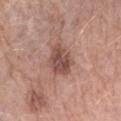Notes:
• workup — total-body-photography surveillance lesion; no biopsy
• illumination — white-light
• automated metrics — a lesion area of about 9 mm², an eccentricity of roughly 0.55, and two-axis asymmetry of about 0.2; a border-irregularity rating of about 2/10 and a color-variation rating of about 3.5/10; a lesion-detection confidence of about 100/100
• lesion diameter — ~4 mm (longest diameter)
• image source — total-body-photography crop, ~15 mm field of view
• body site — the left forearm
• patient — female, aged approximately 70